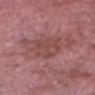notes: imaged on a skin check; not biopsied | imaging modality: 15 mm crop, total-body photography | diameter: ≈5 mm | patient: male, aged approximately 65 | location: the head or neck | illumination: white-light.